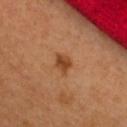Context:
The lesion is on the upper back. Cropped from a total-body skin-imaging series; the visible field is about 15 mm. The lesion's longest dimension is about 2.5 mm. A female patient roughly 35 years of age. Imaged with cross-polarized lighting.